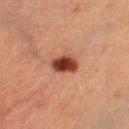Impression:
Captured during whole-body skin photography for melanoma surveillance; the lesion was not biopsied.
Acquisition and patient details:
Cropped from a whole-body photographic skin survey; the tile spans about 15 mm. Located on the left lower leg. Approximately 3.5 mm at its widest. A female subject aged 53–57. Captured under cross-polarized illumination.